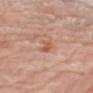Notes:
• biopsy status · total-body-photography surveillance lesion; no biopsy
• imaging modality · ~15 mm tile from a whole-body skin photo
• size · ~3 mm (longest diameter)
• subject · male, aged 78–82
• tile lighting · white-light
• location · the right upper arm